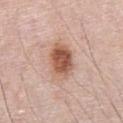Q: Was a biopsy performed?
A: total-body-photography surveillance lesion; no biopsy
Q: Illumination type?
A: white-light
Q: Lesion location?
A: the front of the torso
Q: What is the imaging modality?
A: ~15 mm tile from a whole-body skin photo
Q: What are the patient's age and sex?
A: male, aged 78–82